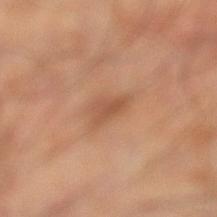This lesion was catalogued during total-body skin photography and was not selected for biopsy. The patient is a male roughly 60 years of age. A close-up tile cropped from a whole-body skin photograph, about 15 mm across. The lesion is on the left lower leg.